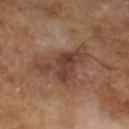Part of a total-body skin-imaging series; this lesion was reviewed on a skin check and was not flagged for biopsy. A male patient, roughly 65 years of age. This image is a 15 mm lesion crop taken from a total-body photograph.